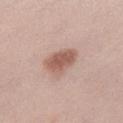* follow-up · imaged on a skin check; not biopsied
* site · the left lower leg
* patient · female, aged 38 to 42
* image source · ~15 mm crop, total-body skin-cancer survey